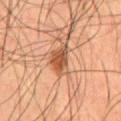Impression:
The lesion was photographed on a routine skin check and not biopsied; there is no pathology result.
Image and clinical context:
The lesion is located on the abdomen. A roughly 15 mm field-of-view crop from a total-body skin photograph. A male subject, aged 53 to 57.